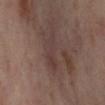Q: Is there a histopathology result?
A: no biopsy performed (imaged during a skin exam)
Q: What did automated image analysis measure?
A: a mean CIELAB color near L≈38 a*≈17 b*≈19, a lesion–skin lightness drop of about 6, and a lesion-to-skin contrast of about 5.5 (normalized; higher = more distinct); a border-irregularity index near 6/10, a color-variation rating of about 2/10, and peripheral color asymmetry of about 0.5
Q: Patient demographics?
A: female, aged approximately 55
Q: How was this image acquired?
A: ~15 mm tile from a whole-body skin photo
Q: Lesion location?
A: the right lower leg